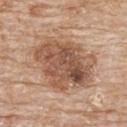No biopsy was performed on this lesion — it was imaged during a full skin examination and was not determined to be concerning. The lesion is located on the upper back. A female subject approximately 85 years of age or older. This image is a 15 mm lesion crop taken from a total-body photograph. This is a white-light tile. The recorded lesion diameter is about 8.5 mm.